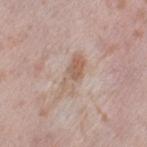Captured during whole-body skin photography for melanoma surveillance; the lesion was not biopsied.
The lesion is on the left thigh.
Cropped from a total-body skin-imaging series; the visible field is about 15 mm.
The tile uses white-light illumination.
A female subject about 40 years old.